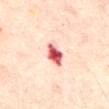– biopsy status: imaged on a skin check; not biopsied
– patient: male, aged 63 to 67
– image source: 15 mm crop, total-body photography
– lesion size: ≈3.5 mm
– tile lighting: cross-polarized illumination
– body site: the front of the torso